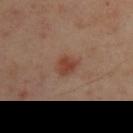Part of a total-body skin-imaging series; this lesion was reviewed on a skin check and was not flagged for biopsy. Measured at roughly 3 mm in maximum diameter. Imaged with cross-polarized lighting. A roughly 15 mm field-of-view crop from a total-body skin photograph. On the left upper arm. A male subject roughly 50 years of age.